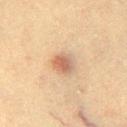* workup: imaged on a skin check; not biopsied
* lighting: cross-polarized illumination
* imaging modality: ~15 mm crop, total-body skin-cancer survey
* lesion diameter: about 3 mm
* patient: female, about 65 years old
* location: the left thigh
* automated lesion analysis: a lesion area of about 6 mm² and a shape-asymmetry score of about 0.2 (0 = symmetric); a within-lesion color-variation index near 4.5/10 and radial color variation of about 1.5